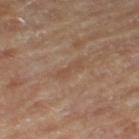<lesion>
<biopsy_status>not biopsied; imaged during a skin examination</biopsy_status>
<site>right thigh</site>
<image>
  <source>total-body photography crop</source>
  <field_of_view_mm>15</field_of_view_mm>
</image>
<patient>
  <sex>male</sex>
  <age_approx>85+</age_approx>
</patient>
<lighting>cross-polarized</lighting>
<lesion_size>
  <long_diameter_mm_approx>3.5</long_diameter_mm_approx>
</lesion_size>
<automated_metrics>
  <eccentricity>0.95</eccentricity>
  <shape_asymmetry>0.35</shape_asymmetry>
  <color_variation_0_10>0.0</color_variation_0_10>
  <peripheral_color_asymmetry>0.0</peripheral_color_asymmetry>
  <nevus_likeness_0_100>0</nevus_likeness_0_100>
</automated_metrics>
</lesion>